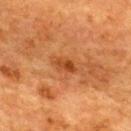- workup · catalogued during a skin exam; not biopsied
- tile lighting · cross-polarized
- lesion diameter · about 2.5 mm
- image source · ~15 mm tile from a whole-body skin photo
- subject · female, in their mid-50s
- body site · the back